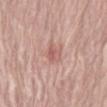Q: Is there a histopathology result?
A: catalogued during a skin exam; not biopsied
Q: What are the patient's age and sex?
A: male, in their 70s
Q: What is the imaging modality?
A: total-body-photography crop, ~15 mm field of view
Q: What did automated image analysis measure?
A: a mean CIELAB color near L≈59 a*≈24 b*≈24, roughly 8 lightness units darker than nearby skin, and a lesion-to-skin contrast of about 5.5 (normalized; higher = more distinct)
Q: What is the lesion's diameter?
A: ~2.5 mm (longest diameter)
Q: Lesion location?
A: the abdomen